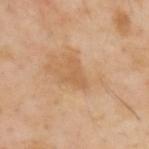Assessment: No biopsy was performed on this lesion — it was imaged during a full skin examination and was not determined to be concerning. Clinical summary: A male patient aged 53–57. Longest diameter approximately 3.5 mm. A 15 mm close-up extracted from a 3D total-body photography capture. Automated image analysis of the tile measured a footprint of about 5 mm², a shape eccentricity near 0.7, and a symmetry-axis asymmetry near 0.6. The software also gave border irregularity of about 6 on a 0–10 scale, a within-lesion color-variation index near 1/10, and a peripheral color-asymmetry measure near 0.5. The analysis additionally found a nevus-likeness score of about 0/100 and a lesion-detection confidence of about 100/100. The lesion is on the upper back. Captured under cross-polarized illumination.